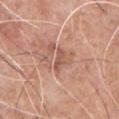A 15 mm crop from a total-body photograph taken for skin-cancer surveillance.
The tile uses white-light illumination.
A male patient aged approximately 60.
The lesion is located on the chest.